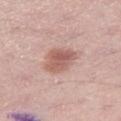Findings:
– workup — total-body-photography surveillance lesion; no biopsy
– image — 15 mm crop, total-body photography
– illumination — white-light illumination
– lesion size — ≈4 mm
– anatomic site — the leg
– subject — male, roughly 50 years of age